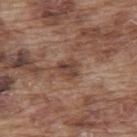{"biopsy_status": "not biopsied; imaged during a skin examination", "patient": {"sex": "male", "age_approx": 75}, "lighting": "white-light", "automated_metrics": {"cielab_L": 42, "cielab_a": 19, "cielab_b": 26, "vs_skin_darker_L": 9.0, "vs_skin_contrast_norm": 7.5}, "image": {"source": "total-body photography crop", "field_of_view_mm": 15}, "site": "upper back", "lesion_size": {"long_diameter_mm_approx": 2.5}}